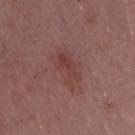Clinical impression:
No biopsy was performed on this lesion — it was imaged during a full skin examination and was not determined to be concerning.
Image and clinical context:
Cropped from a total-body skin-imaging series; the visible field is about 15 mm. The patient is a female aged 43–47. Captured under white-light illumination. Automated image analysis of the tile measured a lesion area of about 9.5 mm² and an outline eccentricity of about 0.85 (0 = round, 1 = elongated). It also reported an average lesion color of about L≈41 a*≈23 b*≈22 (CIELAB), about 6 CIELAB-L* units darker than the surrounding skin, and a lesion-to-skin contrast of about 5.5 (normalized; higher = more distinct). The lesion is on the arm.